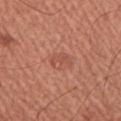| key | value |
|---|---|
| biopsy status | imaged on a skin check; not biopsied |
| acquisition | total-body-photography crop, ~15 mm field of view |
| tile lighting | white-light |
| subject | male, aged 63–67 |
| body site | the arm |
| lesion size | ≈2.5 mm |